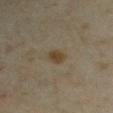notes=no biopsy performed (imaged during a skin exam).